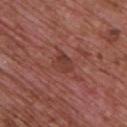Impression: This lesion was catalogued during total-body skin photography and was not selected for biopsy.